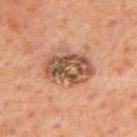{
  "biopsy_status": "not biopsied; imaged during a skin examination",
  "patient": {
    "sex": "male",
    "age_approx": 60
  },
  "image": {
    "source": "total-body photography crop",
    "field_of_view_mm": 15
  },
  "lesion_size": {
    "long_diameter_mm_approx": 5.5
  },
  "site": "mid back",
  "automated_metrics": {
    "cielab_L": 39,
    "cielab_a": 16,
    "cielab_b": 25,
    "vs_skin_darker_L": 10.0,
    "vs_skin_contrast_norm": 9.0,
    "nevus_likeness_0_100": 5,
    "lesion_detection_confidence_0_100": 100
  },
  "lighting": "cross-polarized"
}